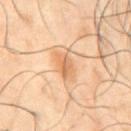Q: Is there a histopathology result?
A: catalogued during a skin exam; not biopsied
Q: What is the imaging modality?
A: total-body-photography crop, ~15 mm field of view
Q: What did automated image analysis measure?
A: a lesion color around L≈51 a*≈18 b*≈31 in CIELAB and roughly 8 lightness units darker than nearby skin; an automated nevus-likeness rating near 95 out of 100 and a detector confidence of about 100 out of 100 that the crop contains a lesion
Q: Lesion location?
A: the abdomen
Q: What are the patient's age and sex?
A: male, about 50 years old
Q: What lighting was used for the tile?
A: cross-polarized illumination